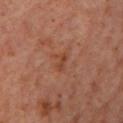{"biopsy_status": "not biopsied; imaged during a skin examination", "automated_metrics": {"color_variation_0_10": 0.0, "peripheral_color_asymmetry": 0.0}, "image": {"source": "total-body photography crop", "field_of_view_mm": 15}, "site": "left lower leg", "lesion_size": {"long_diameter_mm_approx": 2.5}, "patient": {"sex": "male", "age_approx": 60}}